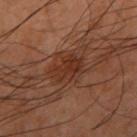{"biopsy_status": "not biopsied; imaged during a skin examination", "site": "right upper arm", "lighting": "cross-polarized", "image": {"source": "total-body photography crop", "field_of_view_mm": 15}, "patient": {"sex": "male", "age_approx": 45}}